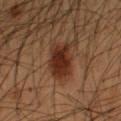{"biopsy_status": "not biopsied; imaged during a skin examination", "patient": {"sex": "male", "age_approx": 55}, "lighting": "cross-polarized", "image": {"source": "total-body photography crop", "field_of_view_mm": 15}, "site": "arm"}